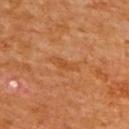Clinical impression:
The lesion was photographed on a routine skin check and not biopsied; there is no pathology result.
Clinical summary:
The recorded lesion diameter is about 3.5 mm. The subject is a male about 65 years old. Automated tile analysis of the lesion measured a shape eccentricity near 0.95 and two-axis asymmetry of about 0.45. And it measured an average lesion color of about L≈48 a*≈26 b*≈40 (CIELAB), a lesion–skin lightness drop of about 6, and a lesion-to-skin contrast of about 5.5 (normalized; higher = more distinct). Imaged with cross-polarized lighting. From the upper back. A 15 mm close-up extracted from a 3D total-body photography capture.